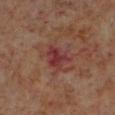No biopsy was performed on this lesion — it was imaged during a full skin examination and was not determined to be concerning.